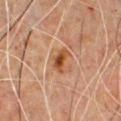Notes:
* notes · no biopsy performed (imaged during a skin exam)
* subject · male, roughly 50 years of age
* image · total-body-photography crop, ~15 mm field of view
* lighting · cross-polarized illumination
* location · the chest
* lesion diameter · ~3 mm (longest diameter)